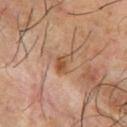Assessment:
The lesion was photographed on a routine skin check and not biopsied; there is no pathology result.
Acquisition and patient details:
A 15 mm close-up tile from a total-body photography series done for melanoma screening. This is a cross-polarized tile. The patient is a male in their mid-60s. On the upper back.